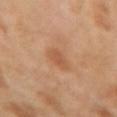site = the left forearm | acquisition = ~15 mm tile from a whole-body skin photo | illumination = cross-polarized illumination | TBP lesion metrics = a mean CIELAB color near L≈56 a*≈22 b*≈36 and a normalized lesion–skin contrast near 5.5; a detector confidence of about 100 out of 100 that the crop contains a lesion | patient = female, in their 40s | size = ~2.5 mm (longest diameter).